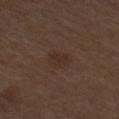The lesion was photographed on a routine skin check and not biopsied; there is no pathology result.
Longest diameter approximately 3 mm.
An algorithmic analysis of the crop reported a lesion area of about 5.5 mm² and two-axis asymmetry of about 0.2. The software also gave a lesion color around L≈29 a*≈15 b*≈22 in CIELAB. The analysis additionally found border irregularity of about 2 on a 0–10 scale, a color-variation rating of about 1.5/10, and peripheral color asymmetry of about 0.5. And it measured a lesion-detection confidence of about 100/100.
This image is a 15 mm lesion crop taken from a total-body photograph.
A male patient, in their mid-70s.
The lesion is on the leg.